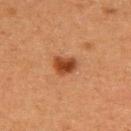Clinical impression:
Imaged during a routine full-body skin examination; the lesion was not biopsied and no histopathology is available.
Background:
Cropped from a whole-body photographic skin survey; the tile spans about 15 mm. A female subject, aged 38–42. This is a cross-polarized tile. Located on the upper back.